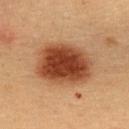Q: Was a biopsy performed?
A: no biopsy performed (imaged during a skin exam)
Q: How was this image acquired?
A: ~15 mm crop, total-body skin-cancer survey
Q: Lesion size?
A: about 6.5 mm
Q: Who is the patient?
A: female, roughly 30 years of age
Q: Where on the body is the lesion?
A: the upper back
Q: Illumination type?
A: cross-polarized